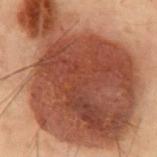Notes:
* lesion size · about 15.5 mm
* patient · male, in their mid-50s
* body site · the abdomen
* automated metrics · a symmetry-axis asymmetry near 0.4; a lesion color around L≈37 a*≈22 b*≈26 in CIELAB, roughly 17 lightness units darker than nearby skin, and a lesion-to-skin contrast of about 13.5 (normalized; higher = more distinct); a nevus-likeness score of about 65/100
* acquisition · 15 mm crop, total-body photography